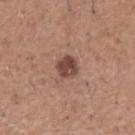Captured during whole-body skin photography for melanoma surveillance; the lesion was not biopsied. A male subject aged approximately 50. The recorded lesion diameter is about 2.5 mm. A close-up tile cropped from a whole-body skin photograph, about 15 mm across. Located on the right upper arm. The tile uses white-light illumination.